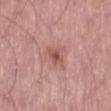lighting: white-light illumination | subject: male, roughly 55 years of age | body site: the right thigh | image-analysis metrics: an outline eccentricity of about 0.7 (0 = round, 1 = elongated); about 9 CIELAB-L* units darker than the surrounding skin and a lesion-to-skin contrast of about 6.5 (normalized; higher = more distinct); border irregularity of about 3 on a 0–10 scale and internal color variation of about 4.5 on a 0–10 scale | acquisition: ~15 mm tile from a whole-body skin photo.